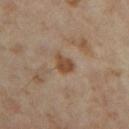<lesion>
<biopsy_status>not biopsied; imaged during a skin examination</biopsy_status>
<automated_metrics>
  <eccentricity>0.7</eccentricity>
  <border_irregularity_0_10>2.0</border_irregularity_0_10>
  <peripheral_color_asymmetry>1.0</peripheral_color_asymmetry>
  <nevus_likeness_0_100>45</nevus_likeness_0_100>
  <lesion_detection_confidence_0_100>100</lesion_detection_confidence_0_100>
</automated_metrics>
<lighting>cross-polarized</lighting>
<lesion_size>
  <long_diameter_mm_approx>2.5</long_diameter_mm_approx>
</lesion_size>
<image>
  <source>total-body photography crop</source>
  <field_of_view_mm>15</field_of_view_mm>
</image>
<patient>
  <sex>female</sex>
  <age_approx>35</age_approx>
</patient>
<site>left thigh</site>
</lesion>